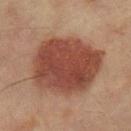Impression: No biopsy was performed on this lesion — it was imaged during a full skin examination and was not determined to be concerning. Image and clinical context: The total-body-photography lesion software estimated a footprint of about 44 mm², an eccentricity of roughly 0.6, and two-axis asymmetry of about 0.15. The analysis additionally found a mean CIELAB color near L≈39 a*≈22 b*≈25 and a lesion–skin lightness drop of about 12. It also reported an automated nevus-likeness rating near 100 out of 100. The lesion is located on the right thigh. The lesion's longest dimension is about 8.5 mm. A 15 mm close-up extracted from a 3D total-body photography capture. A female subject aged 53 to 57.